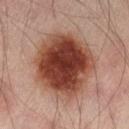{"biopsy_status": "not biopsied; imaged during a skin examination", "site": "leg", "automated_metrics": {"area_mm2_approx": 46.0, "eccentricity": 0.5, "shape_asymmetry": 0.1, "vs_skin_darker_L": 16.0, "vs_skin_contrast_norm": 13.0, "nevus_likeness_0_100": 100, "lesion_detection_confidence_0_100": 100}, "patient": {"sex": "male", "age_approx": 40}, "image": {"source": "total-body photography crop", "field_of_view_mm": 15}}